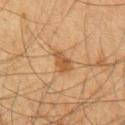Q: Lesion size?
A: ~2.5 mm (longest diameter)
Q: Who is the patient?
A: male, roughly 65 years of age
Q: Where on the body is the lesion?
A: the chest
Q: What is the imaging modality?
A: 15 mm crop, total-body photography